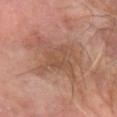Q: Was this lesion biopsied?
A: total-body-photography surveillance lesion; no biopsy
Q: What did automated image analysis measure?
A: a mean CIELAB color near L≈48 a*≈19 b*≈27, roughly 8 lightness units darker than nearby skin, and a normalized border contrast of about 6
Q: What is the anatomic site?
A: the left forearm
Q: What kind of image is this?
A: ~15 mm crop, total-body skin-cancer survey
Q: Patient demographics?
A: male, in their mid- to late 60s
Q: Lesion size?
A: ≈7.5 mm
Q: How was the tile lit?
A: cross-polarized illumination